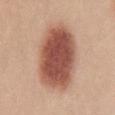  biopsy_status: not biopsied; imaged during a skin examination
  image:
    source: total-body photography crop
    field_of_view_mm: 15
  patient:
    sex: female
    age_approx: 20
  site: mid back
  lighting: white-light